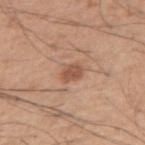Q: Is there a histopathology result?
A: no biopsy performed (imaged during a skin exam)
Q: What did automated image analysis measure?
A: a footprint of about 4.5 mm², an outline eccentricity of about 0.6 (0 = round, 1 = elongated), and a symmetry-axis asymmetry near 0.15; a mean CIELAB color near L≈53 a*≈22 b*≈30, about 10 CIELAB-L* units darker than the surrounding skin, and a normalized lesion–skin contrast near 7; a color-variation rating of about 2.5/10 and a peripheral color-asymmetry measure near 1
Q: What is the anatomic site?
A: the arm
Q: Patient demographics?
A: male, aged 58–62
Q: What kind of image is this?
A: ~15 mm tile from a whole-body skin photo
Q: How large is the lesion?
A: ~2.5 mm (longest diameter)